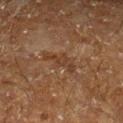Context:
Cropped from a whole-body photographic skin survey; the tile spans about 15 mm. The subject is a male aged around 60. The lesion is on the leg.